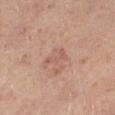This lesion was catalogued during total-body skin photography and was not selected for biopsy. The lesion's longest dimension is about 3 mm. Located on the left lower leg. A roughly 15 mm field-of-view crop from a total-body skin photograph. A male subject, aged around 55.